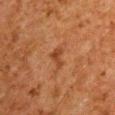Recorded during total-body skin imaging; not selected for excision or biopsy. From the right upper arm. A region of skin cropped from a whole-body photographic capture, roughly 15 mm wide. The subject is a male approximately 60 years of age. The tile uses cross-polarized illumination.